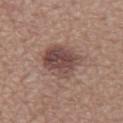This lesion was catalogued during total-body skin photography and was not selected for biopsy. A male subject aged 63–67. Imaged with white-light lighting. The lesion is on the abdomen. A 15 mm close-up extracted from a 3D total-body photography capture.